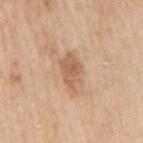Q: Was a biopsy performed?
A: no biopsy performed (imaged during a skin exam)
Q: How was this image acquired?
A: ~15 mm tile from a whole-body skin photo
Q: What lighting was used for the tile?
A: white-light illumination
Q: Lesion location?
A: the right upper arm
Q: Lesion size?
A: ≈4.5 mm
Q: Who is the patient?
A: male, approximately 60 years of age
Q: Automated lesion metrics?
A: two-axis asymmetry of about 0.25